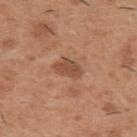{
  "biopsy_status": "not biopsied; imaged during a skin examination",
  "site": "upper back",
  "image": {
    "source": "total-body photography crop",
    "field_of_view_mm": 15
  },
  "patient": {
    "sex": "male",
    "age_approx": 40
  }
}